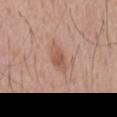Q: Where on the body is the lesion?
A: the mid back
Q: How was this image acquired?
A: 15 mm crop, total-body photography
Q: Patient demographics?
A: male, aged approximately 55